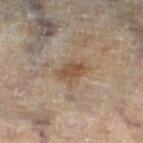{
  "biopsy_status": "not biopsied; imaged during a skin examination",
  "lesion_size": {
    "long_diameter_mm_approx": 3.0
  },
  "site": "left lower leg",
  "image": {
    "source": "total-body photography crop",
    "field_of_view_mm": 15
  },
  "patient": {
    "sex": "female",
    "age_approx": 60
  },
  "automated_metrics": {
    "area_mm2_approx": 5.0,
    "border_irregularity_0_10": 3.0,
    "color_variation_0_10": 3.5,
    "peripheral_color_asymmetry": 1.5,
    "lesion_detection_confidence_0_100": 100
  }
}